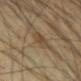location: the left forearm
size: ≈4 mm
tile lighting: cross-polarized
imaging modality: total-body-photography crop, ~15 mm field of view
automated metrics: a lesion area of about 7 mm², an outline eccentricity of about 0.75 (0 = round, 1 = elongated), and two-axis asymmetry of about 0.2; border irregularity of about 2 on a 0–10 scale, a color-variation rating of about 3.5/10, and radial color variation of about 1; a nevus-likeness score of about 20/100 and lesion-presence confidence of about 85/100
subject: male, aged around 65